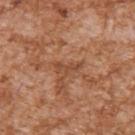No biopsy was performed on this lesion — it was imaged during a full skin examination and was not determined to be concerning. Measured at roughly 3 mm in maximum diameter. Captured under white-light illumination. An algorithmic analysis of the crop reported a lesion area of about 3.5 mm², an outline eccentricity of about 0.9 (0 = round, 1 = elongated), and two-axis asymmetry of about 0.45. The analysis additionally found a border-irregularity rating of about 5.5/10, a within-lesion color-variation index near 0/10, and radial color variation of about 0. A male patient aged 43–47. A 15 mm crop from a total-body photograph taken for skin-cancer surveillance. On the arm.